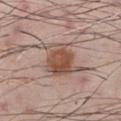{"biopsy_status": "not biopsied; imaged during a skin examination"}